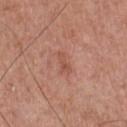biopsy_status: not biopsied; imaged during a skin examination
site: chest
patient:
  sex: male
  age_approx: 70
image:
  source: total-body photography crop
  field_of_view_mm: 15